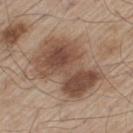Clinical impression:
Imaged during a routine full-body skin examination; the lesion was not biopsied and no histopathology is available.
Context:
The patient is a male about 60 years old. Automated image analysis of the tile measured an area of roughly 36 mm², a shape eccentricity near 0.75, and a symmetry-axis asymmetry near 0.3. The analysis additionally found a border-irregularity index near 4/10 and a within-lesion color-variation index near 7/10. Cropped from a total-body skin-imaging series; the visible field is about 15 mm. The tile uses white-light illumination. Located on the left thigh.